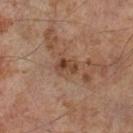<record>
<biopsy_status>not biopsied; imaged during a skin examination</biopsy_status>
<image>
  <source>total-body photography crop</source>
  <field_of_view_mm>15</field_of_view_mm>
</image>
<automated_metrics>
  <area_mm2_approx>4.0</area_mm2_approx>
  <eccentricity>0.75</eccentricity>
  <shape_asymmetry>0.3</shape_asymmetry>
  <cielab_L>40</cielab_L>
  <cielab_a>19</cielab_a>
  <cielab_b>28</cielab_b>
  <vs_skin_darker_L>9.0</vs_skin_darker_L>
  <vs_skin_contrast_norm>8.0</vs_skin_contrast_norm>
  <peripheral_color_asymmetry>3.0</peripheral_color_asymmetry>
</automated_metrics>
<patient>
  <sex>male</sex>
  <age_approx>65</age_approx>
</patient>
<site>left thigh</site>
<lighting>cross-polarized</lighting>
<lesion_size>
  <long_diameter_mm_approx>2.5</long_diameter_mm_approx>
</lesion_size>
</record>